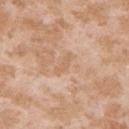<case>
<biopsy_status>not biopsied; imaged during a skin examination</biopsy_status>
<automated_metrics>
  <area_mm2_approx>3.0</area_mm2_approx>
  <eccentricity>0.9</eccentricity>
  <shape_asymmetry>0.4</shape_asymmetry>
  <lesion_detection_confidence_0_100>100</lesion_detection_confidence_0_100>
</automated_metrics>
<patient>
  <sex>female</sex>
  <age_approx>25</age_approx>
</patient>
<lesion_size>
  <long_diameter_mm_approx>3.0</long_diameter_mm_approx>
</lesion_size>
<image>
  <source>total-body photography crop</source>
  <field_of_view_mm>15</field_of_view_mm>
</image>
<site>right upper arm</site>
</case>